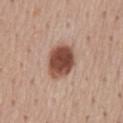Clinical impression: The lesion was photographed on a routine skin check and not biopsied; there is no pathology result. Clinical summary: This image is a 15 mm lesion crop taken from a total-body photograph. A male subject aged approximately 55. The lesion is located on the mid back.